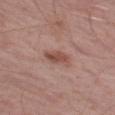Clinical impression:
The lesion was tiled from a total-body skin photograph and was not biopsied.
Context:
From the right thigh. Automated image analysis of the tile measured a lesion–skin lightness drop of about 10 and a normalized lesion–skin contrast near 7.5. A male patient approximately 60 years of age. Cropped from a total-body skin-imaging series; the visible field is about 15 mm.